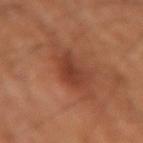This lesion was catalogued during total-body skin photography and was not selected for biopsy. The lesion is on the arm. A region of skin cropped from a whole-body photographic capture, roughly 15 mm wide. Captured under cross-polarized illumination. The recorded lesion diameter is about 4.5 mm. The subject is a male aged 48–52.